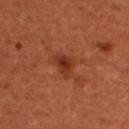Impression: Recorded during total-body skin imaging; not selected for excision or biopsy. Context: A female patient, aged 48 to 52. The lesion's longest dimension is about 3 mm. Imaged with cross-polarized lighting. Cropped from a total-body skin-imaging series; the visible field is about 15 mm.